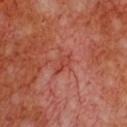The tile uses cross-polarized illumination.
A roughly 15 mm field-of-view crop from a total-body skin photograph.
The total-body-photography lesion software estimated a nevus-likeness score of about 0/100 and a lesion-detection confidence of about 70/100.
Measured at roughly 2.5 mm in maximum diameter.
A male subject, in their mid- to late 50s.
On the chest.